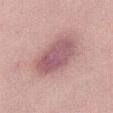The lesion was photographed on a routine skin check and not biopsied; there is no pathology result. The lesion-visualizer software estimated an area of roughly 17 mm², a shape eccentricity near 0.8, and two-axis asymmetry of about 0.15. And it measured a lesion color around L≈56 a*≈24 b*≈17 in CIELAB, roughly 12 lightness units darker than nearby skin, and a lesion-to-skin contrast of about 8 (normalized; higher = more distinct). The analysis additionally found a border-irregularity rating of about 2/10 and internal color variation of about 3.5 on a 0–10 scale. The software also gave a classifier nevus-likeness of about 10/100 and a lesion-detection confidence of about 100/100. The recorded lesion diameter is about 6 mm. The lesion is located on the right thigh. Captured under white-light illumination. A male patient, approximately 40 years of age. This image is a 15 mm lesion crop taken from a total-body photograph.